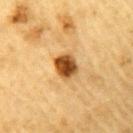No biopsy was performed on this lesion — it was imaged during a full skin examination and was not determined to be concerning.